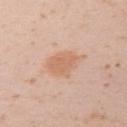| field | value |
|---|---|
| lesion diameter | about 4 mm |
| image | 15 mm crop, total-body photography |
| tile lighting | white-light illumination |
| location | the arm |
| automated metrics | a lesion color around L≈65 a*≈21 b*≈33 in CIELAB, roughly 8 lightness units darker than nearby skin, and a lesion-to-skin contrast of about 6 (normalized; higher = more distinct); a lesion-detection confidence of about 100/100 |
| patient | female, aged around 20 |